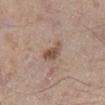The lesion is located on the right lower leg. Approximately 3 mm at its widest. Cropped from a total-body skin-imaging series; the visible field is about 15 mm. This is a white-light tile. A male patient aged approximately 55.